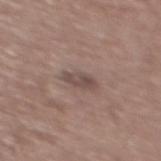Assessment:
Recorded during total-body skin imaging; not selected for excision or biopsy.
Context:
Cropped from a whole-body photographic skin survey; the tile spans about 15 mm. Located on the back. The patient is a male roughly 60 years of age.